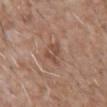This lesion was catalogued during total-body skin photography and was not selected for biopsy. A male patient, aged 58–62. Cropped from a whole-body photographic skin survey; the tile spans about 15 mm. From the chest. Longest diameter approximately 3 mm. The tile uses white-light illumination. Automated image analysis of the tile measured an area of roughly 4 mm², a shape eccentricity near 0.75, and a symmetry-axis asymmetry near 0.45. The software also gave a normalized lesion–skin contrast near 6.5. And it measured an automated nevus-likeness rating near 0 out of 100 and a detector confidence of about 100 out of 100 that the crop contains a lesion.